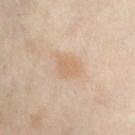A close-up tile cropped from a whole-body skin photograph, about 15 mm across. The lesion is on the abdomen. Automated image analysis of the tile measured a shape eccentricity near 0.7 and two-axis asymmetry of about 0.25. It also reported roughly 7 lightness units darker than nearby skin and a lesion-to-skin contrast of about 5 (normalized; higher = more distinct). The software also gave a border-irregularity index near 2.5/10. A female patient, approximately 55 years of age.